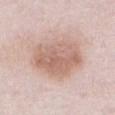Impression:
This lesion was catalogued during total-body skin photography and was not selected for biopsy.
Background:
On the abdomen. A female subject about 65 years old. This is a white-light tile. Measured at roughly 7.5 mm in maximum diameter. Cropped from a whole-body photographic skin survey; the tile spans about 15 mm. The lesion-visualizer software estimated a nevus-likeness score of about 20/100 and a detector confidence of about 100 out of 100 that the crop contains a lesion.